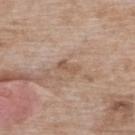Q: Was this lesion biopsied?
A: no biopsy performed (imaged during a skin exam)
Q: What lighting was used for the tile?
A: white-light illumination
Q: Patient demographics?
A: female, in their mid- to late 70s
Q: How was this image acquired?
A: ~15 mm crop, total-body skin-cancer survey
Q: Lesion location?
A: the upper back